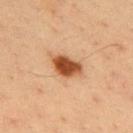Imaged during a routine full-body skin examination; the lesion was not biopsied and no histopathology is available.
This image is a 15 mm lesion crop taken from a total-body photograph.
From the upper back.
Imaged with cross-polarized lighting.
A male subject, roughly 35 years of age.